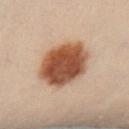Q: Is there a histopathology result?
A: no biopsy performed (imaged during a skin exam)
Q: What kind of image is this?
A: total-body-photography crop, ~15 mm field of view
Q: Where on the body is the lesion?
A: the left leg
Q: What are the patient's age and sex?
A: male, aged around 50
Q: Illumination type?
A: cross-polarized illumination
Q: What is the lesion's diameter?
A: ≈6 mm